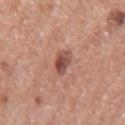Recorded during total-body skin imaging; not selected for excision or biopsy. A region of skin cropped from a whole-body photographic capture, roughly 15 mm wide. On the mid back. A male patient about 75 years old. The recorded lesion diameter is about 2.5 mm. The total-body-photography lesion software estimated an outline eccentricity of about 0.7 (0 = round, 1 = elongated) and two-axis asymmetry of about 0.2. The software also gave a lesion color around L≈49 a*≈24 b*≈25 in CIELAB and roughly 13 lightness units darker than nearby skin. And it measured a border-irregularity index near 2/10, a within-lesion color-variation index near 4.5/10, and a peripheral color-asymmetry measure near 1.5. It also reported a classifier nevus-likeness of about 85/100. The tile uses white-light illumination.